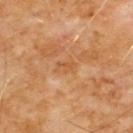Part of a total-body skin-imaging series; this lesion was reviewed on a skin check and was not flagged for biopsy.